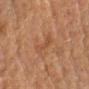biopsy status = catalogued during a skin exam; not biopsied
acquisition = ~15 mm crop, total-body skin-cancer survey
subject = female, aged 48–52
site = the left forearm